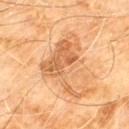Captured during whole-body skin photography for melanoma surveillance; the lesion was not biopsied.
A 15 mm close-up extracted from a 3D total-body photography capture.
From the chest.
Measured at roughly 7.5 mm in maximum diameter.
A male subject aged 58–62.
This is a cross-polarized tile.